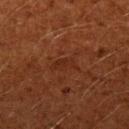Clinical impression: The lesion was tiled from a total-body skin photograph and was not biopsied. Context: Automated image analysis of the tile measured an area of roughly 4.5 mm², a shape eccentricity near 0.9, and two-axis asymmetry of about 0.4. The software also gave an automated nevus-likeness rating near 0 out of 100. A male subject aged 58–62. A lesion tile, about 15 mm wide, cut from a 3D total-body photograph. The lesion is located on the left upper arm. This is a cross-polarized tile. Approximately 3.5 mm at its widest.